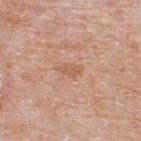Clinical impression: Part of a total-body skin-imaging series; this lesion was reviewed on a skin check and was not flagged for biopsy. Clinical summary: A 15 mm close-up extracted from a 3D total-body photography capture. A male patient aged 73 to 77. Longest diameter approximately 2.5 mm. On the upper back. This is a white-light tile.